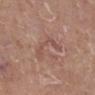Imaged during a routine full-body skin examination; the lesion was not biopsied and no histopathology is available. A 15 mm crop from a total-body photograph taken for skin-cancer surveillance. A female subject, aged around 75. Located on the right lower leg.